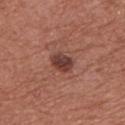An algorithmic analysis of the crop reported a footprint of about 5.5 mm², an outline eccentricity of about 0.65 (0 = round, 1 = elongated), and two-axis asymmetry of about 0.15. And it measured a border-irregularity index near 1.5/10 and radial color variation of about 1.5. The tile uses white-light illumination. The patient is a male aged 73 to 77. A lesion tile, about 15 mm wide, cut from a 3D total-body photograph. The lesion is on the front of the torso.